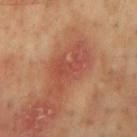On the mid back. A region of skin cropped from a whole-body photographic capture, roughly 15 mm wide. Imaged with cross-polarized lighting. A male patient, about 55 years old.